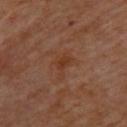Clinical impression: Recorded during total-body skin imaging; not selected for excision or biopsy. Image and clinical context: The subject is a female aged 63 to 67. Approximately 3.5 mm at its widest. From the back. This is a cross-polarized tile. Cropped from a whole-body photographic skin survey; the tile spans about 15 mm. Automated image analysis of the tile measured a mean CIELAB color near L≈36 a*≈22 b*≈30, roughly 5 lightness units darker than nearby skin, and a normalized lesion–skin contrast near 6. And it measured a border-irregularity index near 6.5/10, internal color variation of about 2 on a 0–10 scale, and a peripheral color-asymmetry measure near 0.5.